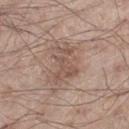Impression: Captured during whole-body skin photography for melanoma surveillance; the lesion was not biopsied. Background: Located on the left thigh. Cropped from a total-body skin-imaging series; the visible field is about 15 mm. The lesion's longest dimension is about 5.5 mm. A male subject aged 53 to 57.